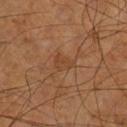No biopsy was performed on this lesion — it was imaged during a full skin examination and was not determined to be concerning.
The subject is a male aged 63 to 67.
The tile uses cross-polarized illumination.
Longest diameter approximately 3 mm.
The lesion is located on the leg.
This image is a 15 mm lesion crop taken from a total-body photograph.